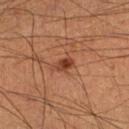Clinical impression:
Captured during whole-body skin photography for melanoma surveillance; the lesion was not biopsied.
Image and clinical context:
The lesion-visualizer software estimated a footprint of about 3.5 mm², an eccentricity of roughly 0.8, and a shape-asymmetry score of about 0.25 (0 = symmetric). It also reported a classifier nevus-likeness of about 90/100. A male subject, aged 58 to 62. The lesion is on the right lower leg. Imaged with cross-polarized lighting. The recorded lesion diameter is about 2.5 mm. A roughly 15 mm field-of-view crop from a total-body skin photograph.